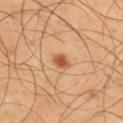| key | value |
|---|---|
| follow-up | catalogued during a skin exam; not biopsied |
| tile lighting | cross-polarized illumination |
| acquisition | ~15 mm crop, total-body skin-cancer survey |
| site | the upper back |
| lesion diameter | ≈2.5 mm |
| subject | male, approximately 45 years of age |
| TBP lesion metrics | border irregularity of about 1.5 on a 0–10 scale and a color-variation rating of about 3/10 |